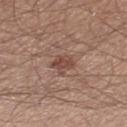Q: Was this lesion biopsied?
A: total-body-photography surveillance lesion; no biopsy
Q: Automated lesion metrics?
A: a normalized border contrast of about 6.5; a border-irregularity rating of about 3.5/10
Q: What is the imaging modality?
A: ~15 mm tile from a whole-body skin photo
Q: What lighting was used for the tile?
A: white-light
Q: Patient demographics?
A: male, about 30 years old
Q: How large is the lesion?
A: ≈3.5 mm
Q: Lesion location?
A: the leg